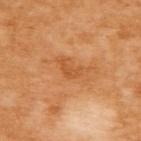Assessment:
Captured during whole-body skin photography for melanoma surveillance; the lesion was not biopsied.
Context:
On the upper back. A female subject, about 55 years old. The total-body-photography lesion software estimated an area of roughly 3.5 mm² and a shape eccentricity near 0.8. It also reported about 7 CIELAB-L* units darker than the surrounding skin. The tile uses cross-polarized illumination. This image is a 15 mm lesion crop taken from a total-body photograph.